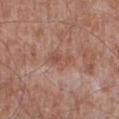notes = imaged on a skin check; not biopsied | anatomic site = the right lower leg | diameter = ≈3 mm | subject = male, roughly 55 years of age | image source = ~15 mm tile from a whole-body skin photo.